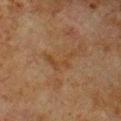notes — catalogued during a skin exam; not biopsied
lesion diameter — about 4 mm
lighting — cross-polarized illumination
site — the chest
image — total-body-photography crop, ~15 mm field of view
subject — male, about 80 years old